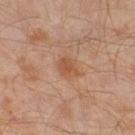Clinical impression:
Part of a total-body skin-imaging series; this lesion was reviewed on a skin check and was not flagged for biopsy.
Background:
This is a cross-polarized tile. From the left leg. A male patient about 30 years old. Cropped from a total-body skin-imaging series; the visible field is about 15 mm.